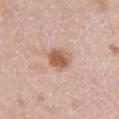Part of a total-body skin-imaging series; this lesion was reviewed on a skin check and was not flagged for biopsy. Located on the left upper arm. An algorithmic analysis of the crop reported a lesion area of about 7 mm², an outline eccentricity of about 0.55 (0 = round, 1 = elongated), and two-axis asymmetry of about 0.15. The analysis additionally found a lesion color around L≈58 a*≈22 b*≈30 in CIELAB, about 13 CIELAB-L* units darker than the surrounding skin, and a normalized border contrast of about 8.5. The software also gave a nevus-likeness score of about 95/100 and a detector confidence of about 100 out of 100 that the crop contains a lesion. A lesion tile, about 15 mm wide, cut from a 3D total-body photograph. Longest diameter approximately 3 mm. The patient is a female aged approximately 50.